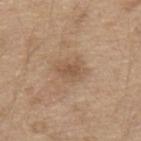notes — no biopsy performed (imaged during a skin exam) | anatomic site — the mid back | lesion diameter — ≈3.5 mm | patient — male, aged around 70 | acquisition — ~15 mm crop, total-body skin-cancer survey | illumination — white-light illumination.